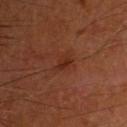No biopsy was performed on this lesion — it was imaged during a full skin examination and was not determined to be concerning. About 3 mm across. Automated image analysis of the tile measured a lesion area of about 4 mm², an outline eccentricity of about 0.8 (0 = round, 1 = elongated), and two-axis asymmetry of about 0.35. Located on the head or neck. This is a cross-polarized tile. Cropped from a total-body skin-imaging series; the visible field is about 15 mm. A male subject, roughly 60 years of age.